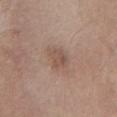Clinical impression: Part of a total-body skin-imaging series; this lesion was reviewed on a skin check and was not flagged for biopsy. Acquisition and patient details: On the chest. A female patient roughly 65 years of age. An algorithmic analysis of the crop reported a footprint of about 5 mm², an outline eccentricity of about 0.8 (0 = round, 1 = elongated), and two-axis asymmetry of about 0.2. It also reported a lesion–skin lightness drop of about 8 and a normalized border contrast of about 6. The software also gave a within-lesion color-variation index near 2.5/10 and peripheral color asymmetry of about 1. And it measured a lesion-detection confidence of about 100/100. Cropped from a whole-body photographic skin survey; the tile spans about 15 mm.